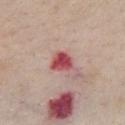| feature | finding |
|---|---|
| size | about 3 mm |
| body site | the front of the torso |
| lighting | white-light illumination |
| patient | female, in their 40s |
| image source | 15 mm crop, total-body photography |
| automated lesion analysis | a border-irregularity rating of about 2/10, a color-variation rating of about 4/10, and radial color variation of about 1; a classifier nevus-likeness of about 0/100 and a lesion-detection confidence of about 100/100 |